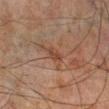Q: Was a biopsy performed?
A: no biopsy performed (imaged during a skin exam)
Q: What are the patient's age and sex?
A: male, about 65 years old
Q: Illumination type?
A: cross-polarized illumination
Q: How was this image acquired?
A: ~15 mm crop, total-body skin-cancer survey
Q: Where on the body is the lesion?
A: the left lower leg
Q: What is the lesion's diameter?
A: ≈3 mm